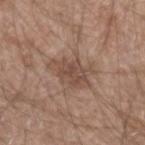notes: total-body-photography surveillance lesion; no biopsy | size: ≈5 mm | image: 15 mm crop, total-body photography | tile lighting: white-light illumination | location: the left forearm | patient: male, aged around 60 | TBP lesion metrics: an average lesion color of about L≈49 a*≈17 b*≈26 (CIELAB), roughly 9 lightness units darker than nearby skin, and a normalized border contrast of about 6.5; a lesion-detection confidence of about 100/100.